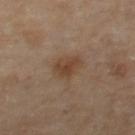Impression: The lesion was tiled from a total-body skin photograph and was not biopsied. Acquisition and patient details: Located on the left thigh. Longest diameter approximately 4 mm. An algorithmic analysis of the crop reported a footprint of about 7 mm², an outline eccentricity of about 0.75 (0 = round, 1 = elongated), and two-axis asymmetry of about 0.3. It also reported a lesion-detection confidence of about 100/100. A 15 mm close-up extracted from a 3D total-body photography capture. A female subject in their 50s.